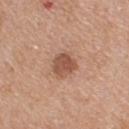The lesion was photographed on a routine skin check and not biopsied; there is no pathology result. Approximately 3 mm at its widest. A male patient aged around 60. From the back. Captured under white-light illumination. A roughly 15 mm field-of-view crop from a total-body skin photograph.